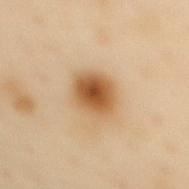Clinical summary:
A female subject, aged 58–62. Located on the back. A 15 mm close-up extracted from a 3D total-body photography capture. An algorithmic analysis of the crop reported a footprint of about 11 mm², an outline eccentricity of about 0.55 (0 = round, 1 = elongated), and two-axis asymmetry of about 0.1. The software also gave a mean CIELAB color near L≈49 a*≈18 b*≈35 and about 13 CIELAB-L* units darker than the surrounding skin. It also reported a nevus-likeness score of about 100/100 and lesion-presence confidence of about 100/100. Captured under cross-polarized illumination. Longest diameter approximately 4 mm.